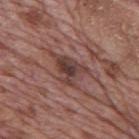| field | value |
|---|---|
| follow-up | catalogued during a skin exam; not biopsied |
| TBP lesion metrics | a shape eccentricity near 0.75 and a shape-asymmetry score of about 0.5 (0 = symmetric); a lesion color around L≈40 a*≈20 b*≈22 in CIELAB, roughly 10 lightness units darker than nearby skin, and a lesion-to-skin contrast of about 8 (normalized; higher = more distinct) |
| image source | 15 mm crop, total-body photography |
| anatomic site | the mid back |
| subject | male, aged approximately 70 |